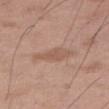Findings:
* workup: total-body-photography surveillance lesion; no biopsy
* lighting: white-light
* imaging modality: 15 mm crop, total-body photography
* body site: the right thigh
* size: ~5 mm (longest diameter)
* patient: male, about 50 years old
* TBP lesion metrics: an eccentricity of roughly 0.9 and a symmetry-axis asymmetry near 0.3; a mean CIELAB color near L≈56 a*≈19 b*≈28, a lesion–skin lightness drop of about 7, and a normalized border contrast of about 5.5; a nevus-likeness score of about 0/100